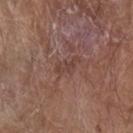Clinical impression: Captured during whole-body skin photography for melanoma surveillance; the lesion was not biopsied. Acquisition and patient details: A male subject in their mid-80s or older. Captured under white-light illumination. An algorithmic analysis of the crop reported a lesion area of about 4 mm² and an outline eccentricity of about 0.9 (0 = round, 1 = elongated). The software also gave a border-irregularity rating of about 5.5/10, a color-variation rating of about 1/10, and peripheral color asymmetry of about 0.5. And it measured a classifier nevus-likeness of about 0/100. The lesion is on the left forearm. A region of skin cropped from a whole-body photographic capture, roughly 15 mm wide.